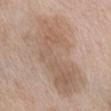– biopsy status · no biopsy performed (imaged during a skin exam)
– body site · the front of the torso
– acquisition · total-body-photography crop, ~15 mm field of view
– tile lighting · white-light
– patient · female, aged around 70
– automated lesion analysis · a footprint of about 55 mm², an eccentricity of roughly 0.9, and two-axis asymmetry of about 0.35; an automated nevus-likeness rating near 0 out of 100
– diameter · ≈12 mm